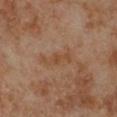Image and clinical context:
Captured under cross-polarized illumination. A male subject about 70 years old. Located on the leg. Cropped from a whole-body photographic skin survey; the tile spans about 15 mm. Approximately 4 mm at its widest.